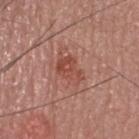Assessment:
Captured during whole-body skin photography for melanoma surveillance; the lesion was not biopsied.
Acquisition and patient details:
Longest diameter approximately 4 mm. This is a white-light tile. A male patient, aged 38 to 42. The total-body-photography lesion software estimated a color-variation rating of about 4/10 and peripheral color asymmetry of about 1.5. From the head or neck. A lesion tile, about 15 mm wide, cut from a 3D total-body photograph.